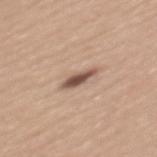Q: Is there a histopathology result?
A: no biopsy performed (imaged during a skin exam)
Q: How was this image acquired?
A: total-body-photography crop, ~15 mm field of view
Q: What did automated image analysis measure?
A: an area of roughly 4.5 mm², a shape eccentricity near 0.9, and a symmetry-axis asymmetry near 0.3; a lesion–skin lightness drop of about 15 and a normalized lesion–skin contrast near 10; a classifier nevus-likeness of about 60/100 and a lesion-detection confidence of about 100/100
Q: Lesion location?
A: the mid back
Q: Patient demographics?
A: female, aged 43–47
Q: What is the lesion's diameter?
A: ~3.5 mm (longest diameter)
Q: What lighting was used for the tile?
A: white-light illumination